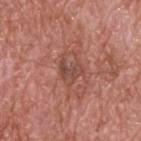Part of a total-body skin-imaging series; this lesion was reviewed on a skin check and was not flagged for biopsy.
Measured at roughly 3 mm in maximum diameter.
The tile uses white-light illumination.
The patient is a male aged around 60.
A roughly 15 mm field-of-view crop from a total-body skin photograph.
On the back.